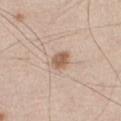<case>
<biopsy_status>not biopsied; imaged during a skin examination</biopsy_status>
<automated_metrics>
  <area_mm2_approx>4.0</area_mm2_approx>
  <eccentricity>0.7</eccentricity>
  <shape_asymmetry>0.25</shape_asymmetry>
  <cielab_L>59</cielab_L>
  <cielab_a>18</cielab_a>
  <cielab_b>30</cielab_b>
  <vs_skin_darker_L>12.0</vs_skin_darker_L>
  <vs_skin_contrast_norm>8.5</vs_skin_contrast_norm>
  <nevus_likeness_0_100>90</nevus_likeness_0_100>
  <lesion_detection_confidence_0_100>100</lesion_detection_confidence_0_100>
</automated_metrics>
<patient>
  <sex>male</sex>
  <age_approx>45</age_approx>
</patient>
<image>
  <source>total-body photography crop</source>
  <field_of_view_mm>15</field_of_view_mm>
</image>
<lighting>white-light</lighting>
<site>chest</site>
</case>